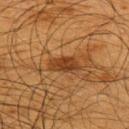patient: male, in their mid- to late 60s; acquisition: ~15 mm tile from a whole-body skin photo; tile lighting: cross-polarized illumination; site: the upper back.